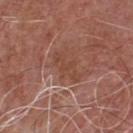Q: What is the anatomic site?
A: the chest
Q: What is the imaging modality?
A: ~15 mm tile from a whole-body skin photo
Q: Automated lesion metrics?
A: a footprint of about 6 mm² and a shape eccentricity near 0.9; a border-irregularity rating of about 5/10 and a color-variation rating of about 1/10; a lesion-detection confidence of about 75/100
Q: What are the patient's age and sex?
A: male, aged around 55
Q: What is the lesion's diameter?
A: ~4 mm (longest diameter)